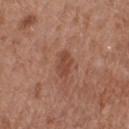Q: Was this lesion biopsied?
A: imaged on a skin check; not biopsied
Q: How large is the lesion?
A: about 3 mm
Q: What is the anatomic site?
A: the right forearm
Q: Illumination type?
A: white-light
Q: How was this image acquired?
A: 15 mm crop, total-body photography
Q: What did automated image analysis measure?
A: a mean CIELAB color near L≈45 a*≈24 b*≈30 and a normalized border contrast of about 6.5; border irregularity of about 2 on a 0–10 scale, a within-lesion color-variation index near 2/10, and peripheral color asymmetry of about 1
Q: Patient demographics?
A: female, aged around 50